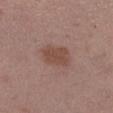The lesion was photographed on a routine skin check and not biopsied; there is no pathology result. From the left thigh. A close-up tile cropped from a whole-body skin photograph, about 15 mm across. Approximately 3.5 mm at its widest. The total-body-photography lesion software estimated a shape eccentricity near 0.7 and two-axis asymmetry of about 0.15. The analysis additionally found a lesion color around L≈47 a*≈20 b*≈25 in CIELAB and a lesion-to-skin contrast of about 7 (normalized; higher = more distinct). Imaged with white-light lighting. The patient is a female in their 40s.